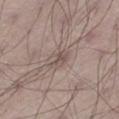Impression: Part of a total-body skin-imaging series; this lesion was reviewed on a skin check and was not flagged for biopsy. Background: A male subject, approximately 70 years of age. An algorithmic analysis of the crop reported a footprint of about 3.5 mm², a shape eccentricity near 0.7, and a shape-asymmetry score of about 0.35 (0 = symmetric). And it measured an average lesion color of about L≈51 a*≈14 b*≈19 (CIELAB) and a normalized lesion–skin contrast near 6. And it measured a border-irregularity index near 3.5/10, a within-lesion color-variation index near 2.5/10, and radial color variation of about 1. The analysis additionally found a classifier nevus-likeness of about 0/100. The lesion is on the leg. A 15 mm crop from a total-body photograph taken for skin-cancer surveillance. This is a white-light tile. The recorded lesion diameter is about 2.5 mm.